This image is a 15 mm lesion crop taken from a total-body photograph; a male patient, aged approximately 25; Automated tile analysis of the lesion measured a lesion area of about 36 mm² and a shape-asymmetry score of about 0.1 (0 = symmetric). It also reported a classifier nevus-likeness of about 80/100 and lesion-presence confidence of about 100/100; on the back; captured under white-light illumination:
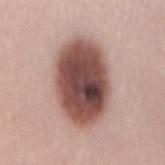histopathology: a dysplastic (Clark) nevus — a benign skin lesion.